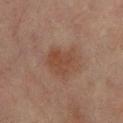About 4 mm across.
From the left lower leg.
The subject is a female roughly 55 years of age.
Cropped from a total-body skin-imaging series; the visible field is about 15 mm.